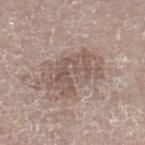Q: Is there a histopathology result?
A: no biopsy performed (imaged during a skin exam)
Q: Where on the body is the lesion?
A: the right lower leg
Q: What is the imaging modality?
A: ~15 mm crop, total-body skin-cancer survey
Q: Patient demographics?
A: male, in their 80s